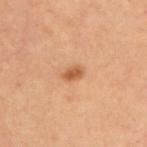The lesion was photographed on a routine skin check and not biopsied; there is no pathology result.